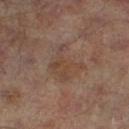{
  "biopsy_status": "not biopsied; imaged during a skin examination",
  "lesion_size": {
    "long_diameter_mm_approx": 4.5
  },
  "site": "leg",
  "lighting": "cross-polarized",
  "image": {
    "source": "total-body photography crop",
    "field_of_view_mm": 15
  },
  "patient": {
    "sex": "male",
    "age_approx": 65
  }
}